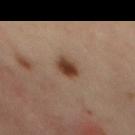Q: Was this lesion biopsied?
A: no biopsy performed (imaged during a skin exam)